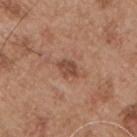Part of a total-body skin-imaging series; this lesion was reviewed on a skin check and was not flagged for biopsy.
The subject is a male aged 53–57.
On the chest.
Cropped from a whole-body photographic skin survey; the tile spans about 15 mm.